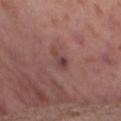Findings:
• biopsy status — imaged on a skin check; not biopsied
• acquisition — total-body-photography crop, ~15 mm field of view
• diameter — ≈3 mm
• patient — female, aged around 55
• tile lighting — cross-polarized
• anatomic site — the left thigh